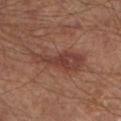follow-up: catalogued during a skin exam; not biopsied | anatomic site: the left lower leg | illumination: cross-polarized illumination | image source: total-body-photography crop, ~15 mm field of view | subject: male, about 65 years old | lesion size: ~7 mm (longest diameter).